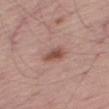Clinical impression: The lesion was tiled from a total-body skin photograph and was not biopsied. Background: Cropped from a whole-body photographic skin survey; the tile spans about 15 mm. Located on the leg. Automated tile analysis of the lesion measured an outline eccentricity of about 0.85 (0 = round, 1 = elongated) and a shape-asymmetry score of about 0.25 (0 = symmetric). It also reported a mean CIELAB color near L≈50 a*≈22 b*≈26 and a lesion–skin lightness drop of about 11. It also reported a border-irregularity index near 2/10, a within-lesion color-variation index near 3/10, and peripheral color asymmetry of about 1. The analysis additionally found a nevus-likeness score of about 85/100 and a lesion-detection confidence of about 100/100. A male subject in their 60s. The tile uses white-light illumination. Approximately 3 mm at its widest.